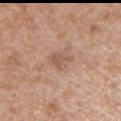- follow-up: catalogued during a skin exam; not biopsied
- automated metrics: an area of roughly 5 mm² and a shape-asymmetry score of about 0.3 (0 = symmetric); an average lesion color of about L≈56 a*≈20 b*≈29 (CIELAB), about 8 CIELAB-L* units darker than the surrounding skin, and a normalized border contrast of about 5.5; border irregularity of about 3.5 on a 0–10 scale, a color-variation rating of about 2/10, and a peripheral color-asymmetry measure near 0.5; a nevus-likeness score of about 0/100 and a lesion-detection confidence of about 100/100
- acquisition: 15 mm crop, total-body photography
- size: ~3 mm (longest diameter)
- lighting: white-light
- anatomic site: the left upper arm
- subject: male, aged 48–52